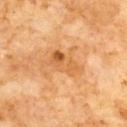No biopsy was performed on this lesion — it was imaged during a full skin examination and was not determined to be concerning. A 15 mm close-up extracted from a 3D total-body photography capture. A male patient, aged approximately 60. The lesion is located on the front of the torso. About 4.5 mm across. Captured under cross-polarized illumination.